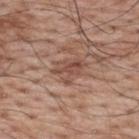Captured during whole-body skin photography for melanoma surveillance; the lesion was not biopsied.
An algorithmic analysis of the crop reported a mean CIELAB color near L≈49 a*≈21 b*≈27 and a normalized lesion–skin contrast near 6.5. The analysis additionally found a nevus-likeness score of about 0/100 and a detector confidence of about 80 out of 100 that the crop contains a lesion.
A region of skin cropped from a whole-body photographic capture, roughly 15 mm wide.
From the upper back.
Imaged with white-light lighting.
The patient is a male aged 68–72.